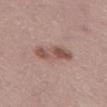| field | value |
|---|---|
| anatomic site | the lower back |
| image | ~15 mm crop, total-body skin-cancer survey |
| subject | male, aged approximately 65 |
| lesion size | ≈5 mm |
| automated lesion analysis | a footprint of about 8.5 mm² and an outline eccentricity of about 0.9 (0 = round, 1 = elongated); a color-variation rating of about 5.5/10 and radial color variation of about 2 |
| lighting | white-light |